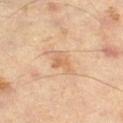Assessment: Part of a total-body skin-imaging series; this lesion was reviewed on a skin check and was not flagged for biopsy. Context: A male subject aged around 60. Located on the left thigh. This image is a 15 mm lesion crop taken from a total-body photograph.